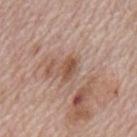Impression:
Part of a total-body skin-imaging series; this lesion was reviewed on a skin check and was not flagged for biopsy.
Acquisition and patient details:
Located on the mid back. The total-body-photography lesion software estimated an average lesion color of about L≈52 a*≈20 b*≈29 (CIELAB) and roughly 9 lightness units darker than nearby skin. And it measured a nevus-likeness score of about 0/100 and lesion-presence confidence of about 100/100. The patient is a male aged around 70. The tile uses white-light illumination. A close-up tile cropped from a whole-body skin photograph, about 15 mm across.